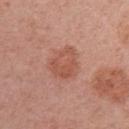notes — no biopsy performed (imaged during a skin exam)
lesion diameter — about 4 mm
body site — the right upper arm
lighting — white-light
subject — female, in their mid-50s
TBP lesion metrics — an area of roughly 11 mm² and an outline eccentricity of about 0.55 (0 = round, 1 = elongated); a lesion color around L≈54 a*≈25 b*≈30 in CIELAB, a lesion–skin lightness drop of about 8, and a normalized lesion–skin contrast near 6; border irregularity of about 2.5 on a 0–10 scale, internal color variation of about 3.5 on a 0–10 scale, and radial color variation of about 1.5
imaging modality — total-body-photography crop, ~15 mm field of view